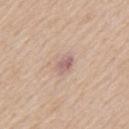workup = total-body-photography surveillance lesion; no biopsy
location = the mid back
patient = male, aged approximately 60
illumination = white-light illumination
acquisition = ~15 mm tile from a whole-body skin photo
lesion diameter = about 2.5 mm